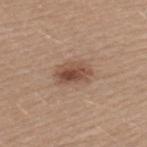The lesion was photographed on a routine skin check and not biopsied; there is no pathology result. Automated tile analysis of the lesion measured a mean CIELAB color near L≈49 a*≈19 b*≈28, roughly 11 lightness units darker than nearby skin, and a lesion-to-skin contrast of about 8.5 (normalized; higher = more distinct). The analysis additionally found border irregularity of about 2 on a 0–10 scale, internal color variation of about 6 on a 0–10 scale, and a peripheral color-asymmetry measure near 2. The analysis additionally found a detector confidence of about 100 out of 100 that the crop contains a lesion. From the upper back. A male subject, in their 40s. A 15 mm close-up tile from a total-body photography series done for melanoma screening. About 4 mm across.